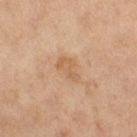biopsy_status: not biopsied; imaged during a skin examination
site: right thigh
lesion_size:
  long_diameter_mm_approx: 3.5
image:
  source: total-body photography crop
  field_of_view_mm: 15
automated_metrics:
  nevus_likeness_0_100: 0
  lesion_detection_confidence_0_100: 100
patient:
  sex: female
  age_approx: 60